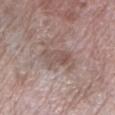Q: Was this lesion biopsied?
A: imaged on a skin check; not biopsied
Q: What are the patient's age and sex?
A: female, about 70 years old
Q: What kind of image is this?
A: ~15 mm tile from a whole-body skin photo
Q: How large is the lesion?
A: ~3.5 mm (longest diameter)
Q: Illumination type?
A: white-light
Q: Lesion location?
A: the leg
Q: What did automated image analysis measure?
A: a lesion color around L≈52 a*≈16 b*≈21 in CIELAB, about 7 CIELAB-L* units darker than the surrounding skin, and a normalized lesion–skin contrast near 5.5